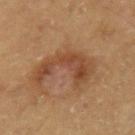Part of a total-body skin-imaging series; this lesion was reviewed on a skin check and was not flagged for biopsy.
The lesion's longest dimension is about 5.5 mm.
Captured under cross-polarized illumination.
A 15 mm close-up tile from a total-body photography series done for melanoma screening.
Located on the left upper arm.
Automated tile analysis of the lesion measured an average lesion color of about L≈39 a*≈19 b*≈29 (CIELAB), a lesion–skin lightness drop of about 8, and a lesion-to-skin contrast of about 7 (normalized; higher = more distinct). It also reported a classifier nevus-likeness of about 5/100 and a lesion-detection confidence of about 100/100.
A male subject, in their 60s.